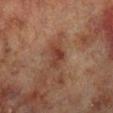Measured at roughly 3 mm in maximum diameter.
This is a cross-polarized tile.
A male patient, aged 68 to 72.
On the right lower leg.
A 15 mm crop from a total-body photograph taken for skin-cancer surveillance.